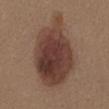  biopsy_status: not biopsied; imaged during a skin examination
  patient:
    sex: female
    age_approx: 40
  lesion_size:
    long_diameter_mm_approx: 10.0
  site: abdomen
  image:
    source: total-body photography crop
    field_of_view_mm: 15